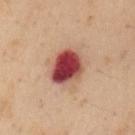<lesion>
<patient>
  <sex>male</sex>
  <age_approx>55</age_approx>
</patient>
<site>left upper arm</site>
<image>
  <source>total-body photography crop</source>
  <field_of_view_mm>15</field_of_view_mm>
</image>
<lighting>cross-polarized</lighting>
</lesion>